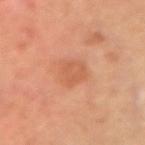{"biopsy_status": "not biopsied; imaged during a skin examination", "image": {"source": "total-body photography crop", "field_of_view_mm": 15}, "patient": {"sex": "male", "age_approx": 65}, "site": "right upper arm", "lighting": "cross-polarized"}